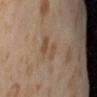Q: Was this lesion biopsied?
A: total-body-photography surveillance lesion; no biopsy
Q: Patient demographics?
A: female, aged 53–57
Q: Illumination type?
A: cross-polarized
Q: Lesion location?
A: the abdomen
Q: What kind of image is this?
A: total-body-photography crop, ~15 mm field of view
Q: Lesion size?
A: ~3.5 mm (longest diameter)